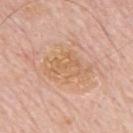Part of a total-body skin-imaging series; this lesion was reviewed on a skin check and was not flagged for biopsy. From the mid back. The recorded lesion diameter is about 5.5 mm. The subject is a male aged approximately 80. Cropped from a total-body skin-imaging series; the visible field is about 15 mm.